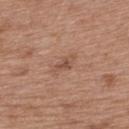workup — no biopsy performed (imaged during a skin exam) | TBP lesion metrics — a lesion color around L≈52 a*≈20 b*≈29 in CIELAB, about 8 CIELAB-L* units darker than the surrounding skin, and a normalized border contrast of about 5.5; a classifier nevus-likeness of about 0/100 and a lesion-detection confidence of about 100/100 | subject — female, aged 38–42 | diameter — about 3.5 mm | location — the back | imaging modality — 15 mm crop, total-body photography.